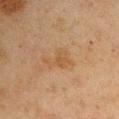Assessment:
This lesion was catalogued during total-body skin photography and was not selected for biopsy.
Acquisition and patient details:
About 4 mm across. The lesion is on the right upper arm. A lesion tile, about 15 mm wide, cut from a 3D total-body photograph. The tile uses cross-polarized illumination. The total-body-photography lesion software estimated a lesion area of about 5.5 mm², an eccentricity of roughly 0.7, and two-axis asymmetry of about 0.6. The analysis additionally found a border-irregularity rating of about 7/10, internal color variation of about 1 on a 0–10 scale, and radial color variation of about 0.5. A male subject aged approximately 45.